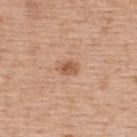- lesion diameter — ~2.5 mm (longest diameter)
- patient — female, about 65 years old
- body site — the back
- acquisition — 15 mm crop, total-body photography